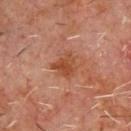A 15 mm crop from a total-body photograph taken for skin-cancer surveillance. About 3 mm across. The tile uses cross-polarized illumination. From the chest. The patient is a male aged approximately 60.